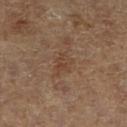Notes:
• lighting: cross-polarized
• image source: ~15 mm tile from a whole-body skin photo
• diameter: about 3 mm
• site: the right lower leg
• subject: male, aged around 85 or older